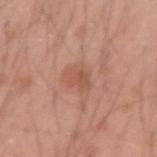workup: imaged on a skin check; not biopsied
subject: male, approximately 20 years of age
lesion size: about 3 mm
lighting: white-light illumination
image source: ~15 mm tile from a whole-body skin photo
site: the left forearm